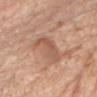Clinical impression:
The lesion was tiled from a total-body skin photograph and was not biopsied.